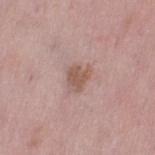This lesion was catalogued during total-body skin photography and was not selected for biopsy. Approximately 3 mm at its widest. A roughly 15 mm field-of-view crop from a total-body skin photograph. A female patient, aged 48 to 52. The lesion is located on the left thigh. The tile uses white-light illumination.